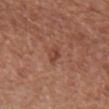<case>
<biopsy_status>not biopsied; imaged during a skin examination</biopsy_status>
<lighting>white-light</lighting>
<patient>
  <sex>female</sex>
  <age_approx>75</age_approx>
</patient>
<site>front of the torso</site>
<image>
  <source>total-body photography crop</source>
  <field_of_view_mm>15</field_of_view_mm>
</image>
<lesion_size>
  <long_diameter_mm_approx>3.0</long_diameter_mm_approx>
</lesion_size>
</case>